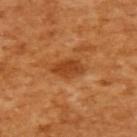| field | value |
|---|---|
| notes | no biopsy performed (imaged during a skin exam) |
| diameter | about 4 mm |
| acquisition | ~15 mm tile from a whole-body skin photo |
| location | the upper back |
| subject | female, aged 53 to 57 |